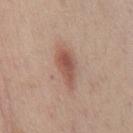| field | value |
|---|---|
| biopsy status | no biopsy performed (imaged during a skin exam) |
| acquisition | 15 mm crop, total-body photography |
| illumination | white-light illumination |
| diameter | ~5 mm (longest diameter) |
| patient | female, in their mid-40s |
| automated metrics | an area of roughly 9 mm², an eccentricity of roughly 0.9, and a symmetry-axis asymmetry near 0.3; a lesion–skin lightness drop of about 11 and a normalized border contrast of about 7.5; a peripheral color-asymmetry measure near 1 |
| site | the chest |